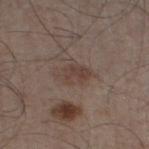Captured during whole-body skin photography for melanoma surveillance; the lesion was not biopsied. The subject is a male aged around 60. The lesion's longest dimension is about 4 mm. The lesion is on the left thigh. Captured under white-light illumination. A lesion tile, about 15 mm wide, cut from a 3D total-body photograph.